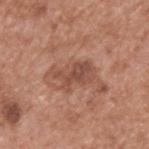Captured during whole-body skin photography for melanoma surveillance; the lesion was not biopsied. On the upper back. A 15 mm close-up extracted from a 3D total-body photography capture. Measured at roughly 5 mm in maximum diameter. The subject is a male aged 63 to 67. The tile uses white-light illumination.